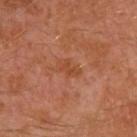{"biopsy_status": "not biopsied; imaged during a skin examination", "site": "left arm", "patient": {"sex": "male", "age_approx": 30}, "image": {"source": "total-body photography crop", "field_of_view_mm": 15}}